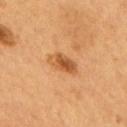The lesion was photographed on a routine skin check and not biopsied; there is no pathology result.
Measured at roughly 4 mm in maximum diameter.
Automated image analysis of the tile measured an area of roughly 6.5 mm² and a shape-asymmetry score of about 0.2 (0 = symmetric). It also reported border irregularity of about 2 on a 0–10 scale and radial color variation of about 2.
The lesion is located on the upper back.
Captured under cross-polarized illumination.
A region of skin cropped from a whole-body photographic capture, roughly 15 mm wide.
A male subject approximately 55 years of age.